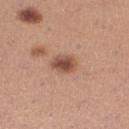follow-up: catalogued during a skin exam; not biopsied | subject: female, roughly 30 years of age | TBP lesion metrics: a classifier nevus-likeness of about 85/100 and a detector confidence of about 100 out of 100 that the crop contains a lesion | image source: total-body-photography crop, ~15 mm field of view | lesion size: ≈3 mm | illumination: white-light | site: the right thigh.